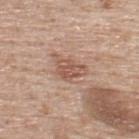| key | value |
|---|---|
| biopsy status | total-body-photography surveillance lesion; no biopsy |
| image-analysis metrics | a lesion color around L≈55 a*≈21 b*≈28 in CIELAB and a normalized border contrast of about 6.5; border irregularity of about 3.5 on a 0–10 scale and a within-lesion color-variation index near 3.5/10; an automated nevus-likeness rating near 0 out of 100 and a lesion-detection confidence of about 100/100 |
| body site | the upper back |
| imaging modality | 15 mm crop, total-body photography |
| illumination | white-light illumination |
| subject | male, aged 73–77 |
| lesion diameter | ≈3.5 mm |